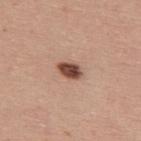Impression:
No biopsy was performed on this lesion — it was imaged during a full skin examination and was not determined to be concerning.
Context:
Approximately 3 mm at its widest. Automated tile analysis of the lesion measured a lesion area of about 4.5 mm², an outline eccentricity of about 0.8 (0 = round, 1 = elongated), and a symmetry-axis asymmetry near 0.25. The software also gave a border-irregularity index near 2/10 and a color-variation rating of about 4/10. Imaged with white-light lighting. A male patient, aged 38 to 42. A 15 mm close-up extracted from a 3D total-body photography capture. Located on the upper back.